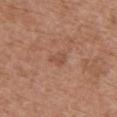Recorded during total-body skin imaging; not selected for excision or biopsy.